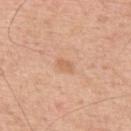This lesion was catalogued during total-body skin photography and was not selected for biopsy. This is a white-light tile. Cropped from a total-body skin-imaging series; the visible field is about 15 mm. On the upper back. A male patient, roughly 55 years of age. Approximately 2.5 mm at its widest.